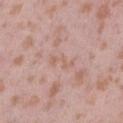Captured during whole-body skin photography for melanoma surveillance; the lesion was not biopsied. Captured under white-light illumination. The lesion's longest dimension is about 3.5 mm. The lesion is located on the right thigh. A close-up tile cropped from a whole-body skin photograph, about 15 mm across. A female patient, aged 38 to 42.